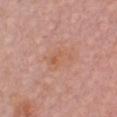Clinical impression: The lesion was photographed on a routine skin check and not biopsied; there is no pathology result. Clinical summary: A female subject aged around 30. A 15 mm close-up extracted from a 3D total-body photography capture. Located on the front of the torso.